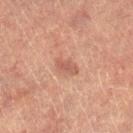This lesion was catalogued during total-body skin photography and was not selected for biopsy.
Automated image analysis of the tile measured a lesion color around L≈47 a*≈21 b*≈25 in CIELAB, roughly 7 lightness units darker than nearby skin, and a normalized lesion–skin contrast near 5.5. And it measured a classifier nevus-likeness of about 5/100 and a lesion-detection confidence of about 100/100.
Captured under cross-polarized illumination.
The lesion is on the right lower leg.
A 15 mm close-up tile from a total-body photography series done for melanoma screening.
A female patient, approximately 70 years of age.
About 3 mm across.